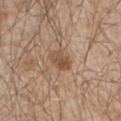notes: imaged on a skin check; not biopsied
image source: total-body-photography crop, ~15 mm field of view
patient: male, roughly 65 years of age
body site: the left upper arm
image-analysis metrics: two-axis asymmetry of about 0.2; a mean CIELAB color near L≈51 a*≈18 b*≈30, about 9 CIELAB-L* units darker than the surrounding skin, and a normalized border contrast of about 7
size: about 3 mm